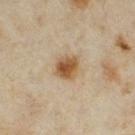Recorded during total-body skin imaging; not selected for excision or biopsy. The lesion's longest dimension is about 3 mm. A female subject, aged around 35. The lesion is located on the leg. An algorithmic analysis of the crop reported roughly 12 lightness units darker than nearby skin. The analysis additionally found border irregularity of about 1.5 on a 0–10 scale, a within-lesion color-variation index near 5.5/10, and peripheral color asymmetry of about 1.5. And it measured a classifier nevus-likeness of about 100/100. A 15 mm close-up tile from a total-body photography series done for melanoma screening. Imaged with cross-polarized lighting.